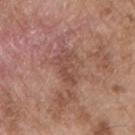This lesion was catalogued during total-body skin photography and was not selected for biopsy. Cropped from a whole-body photographic skin survey; the tile spans about 15 mm. A male subject aged 53 to 57. Automated tile analysis of the lesion measured a shape eccentricity near 0.9. It also reported an average lesion color of about L≈48 a*≈21 b*≈26 (CIELAB) and a normalized lesion–skin contrast near 5.5. And it measured a nevus-likeness score of about 0/100 and lesion-presence confidence of about 95/100. About 4 mm across. On the chest. The tile uses white-light illumination.